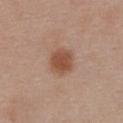notes = catalogued during a skin exam; not biopsied | illumination = white-light illumination | image source = 15 mm crop, total-body photography | subject = female, about 55 years old | anatomic site = the chest | lesion diameter = ≈3 mm.